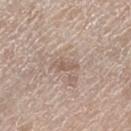<tbp_lesion>
  <biopsy_status>not biopsied; imaged during a skin examination</biopsy_status>
  <automated_metrics>
    <area_mm2_approx>4.0</area_mm2_approx>
    <eccentricity>0.85</eccentricity>
    <shape_asymmetry>0.3</shape_asymmetry>
    <border_irregularity_0_10>3.0</border_irregularity_0_10>
    <color_variation_0_10>1.5</color_variation_0_10>
    <peripheral_color_asymmetry>0.5</peripheral_color_asymmetry>
  </automated_metrics>
  <lighting>white-light</lighting>
  <patient>
    <sex>male</sex>
    <age_approx>80</age_approx>
  </patient>
  <lesion_size>
    <long_diameter_mm_approx>3.0</long_diameter_mm_approx>
  </lesion_size>
  <site>left thigh</site>
  <image>
    <source>total-body photography crop</source>
    <field_of_view_mm>15</field_of_view_mm>
  </image>
</tbp_lesion>